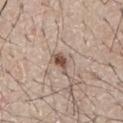Clinical impression:
The lesion was photographed on a routine skin check and not biopsied; there is no pathology result.
Image and clinical context:
Cropped from a whole-body photographic skin survey; the tile spans about 15 mm. The lesion-visualizer software estimated an area of roughly 3 mm², a shape eccentricity near 0.8, and a symmetry-axis asymmetry near 0.4. And it measured a lesion color around L≈51 a*≈17 b*≈25 in CIELAB, about 14 CIELAB-L* units darker than the surrounding skin, and a normalized lesion–skin contrast near 10. The analysis additionally found a nevus-likeness score of about 95/100. Captured under white-light illumination. On the abdomen. Longest diameter approximately 2.5 mm. A male subject, about 65 years old.